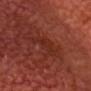{
  "biopsy_status": "not biopsied; imaged during a skin examination",
  "patient": {
    "age_approx": 65
  },
  "lesion_size": {
    "long_diameter_mm_approx": 4.5
  },
  "image": {
    "source": "total-body photography crop",
    "field_of_view_mm": 15
  },
  "lighting": "cross-polarized",
  "site": "head or neck",
  "automated_metrics": {
    "area_mm2_approx": 6.5,
    "shape_asymmetry": 0.5,
    "vs_skin_darker_L": 5.0,
    "vs_skin_contrast_norm": 5.0,
    "peripheral_color_asymmetry": 0.5,
    "nevus_likeness_0_100": 0,
    "lesion_detection_confidence_0_100": 75
  }
}